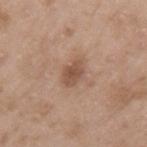follow-up — catalogued during a skin exam; not biopsied
body site — the left upper arm
illumination — white-light
acquisition — total-body-photography crop, ~15 mm field of view
patient — male, aged 48–52
automated lesion analysis — a footprint of about 4.5 mm² and two-axis asymmetry of about 0.25; an average lesion color of about L≈51 a*≈19 b*≈29 (CIELAB), about 10 CIELAB-L* units darker than the surrounding skin, and a normalized border contrast of about 7.5; a classifier nevus-likeness of about 20/100 and a lesion-detection confidence of about 100/100